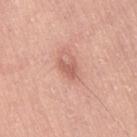<record>
<biopsy_status>not biopsied; imaged during a skin examination</biopsy_status>
<lighting>white-light</lighting>
<site>right thigh</site>
<patient>
  <sex>female</sex>
  <age_approx>40</age_approx>
</patient>
<automated_metrics>
  <eccentricity>0.8</eccentricity>
  <shape_asymmetry>0.45</shape_asymmetry>
  <cielab_L>60</cielab_L>
  <cielab_a>26</cielab_a>
  <cielab_b>28</cielab_b>
  <vs_skin_darker_L>10.0</vs_skin_darker_L>
  <vs_skin_contrast_norm>6.0</vs_skin_contrast_norm>
  <border_irregularity_0_10>4.0</border_irregularity_0_10>
  <color_variation_0_10>0.5</color_variation_0_10>
  <nevus_likeness_0_100>0</nevus_likeness_0_100>
  <lesion_detection_confidence_0_100>100</lesion_detection_confidence_0_100>
</automated_metrics>
<lesion_size>
  <long_diameter_mm_approx>2.5</long_diameter_mm_approx>
</lesion_size>
<image>
  <source>total-body photography crop</source>
  <field_of_view_mm>15</field_of_view_mm>
</image>
</record>